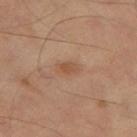  biopsy_status: not biopsied; imaged during a skin examination
  lesion_size:
    long_diameter_mm_approx: 2.5
  image:
    source: total-body photography crop
    field_of_view_mm: 15
  patient:
    sex: male
    age_approx: 65
  lighting: cross-polarized
  automated_metrics:
    area_mm2_approx: 3.5
    eccentricity: 0.85
    cielab_L: 49
    cielab_a: 19
    cielab_b: 31
    vs_skin_darker_L: 7.0
    vs_skin_contrast_norm: 6.0
    nevus_likeness_0_100: 5
  site: left thigh